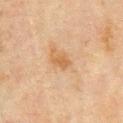{
  "biopsy_status": "not biopsied; imaged during a skin examination",
  "image": {
    "source": "total-body photography crop",
    "field_of_view_mm": 15
  },
  "patient": {
    "sex": "male",
    "age_approx": 70
  },
  "site": "chest"
}